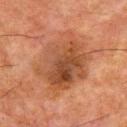Impression:
Imaged during a routine full-body skin examination; the lesion was not biopsied and no histopathology is available.
Context:
Located on the upper back. An algorithmic analysis of the crop reported an area of roughly 34 mm², an outline eccentricity of about 0.7 (0 = round, 1 = elongated), and a shape-asymmetry score of about 0.2 (0 = symmetric). The software also gave a classifier nevus-likeness of about 5/100. A male patient in their mid-60s. A region of skin cropped from a whole-body photographic capture, roughly 15 mm wide.